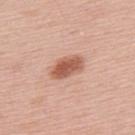Q: What is the lesion's diameter?
A: about 4 mm
Q: Patient demographics?
A: male, in their mid-50s
Q: How was this image acquired?
A: total-body-photography crop, ~15 mm field of view
Q: Where on the body is the lesion?
A: the upper back